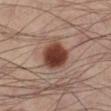This lesion was catalogued during total-body skin photography and was not selected for biopsy. The lesion-visualizer software estimated about 16 CIELAB-L* units darker than the surrounding skin and a normalized border contrast of about 13. It also reported an automated nevus-likeness rating near 100 out of 100 and lesion-presence confidence of about 100/100. The subject is a male about 40 years old. The lesion's longest dimension is about 3.5 mm. The tile uses cross-polarized illumination. From the left lower leg. Cropped from a whole-body photographic skin survey; the tile spans about 15 mm.